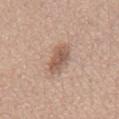subject = male, approximately 70 years of age | diameter = ~4.5 mm (longest diameter) | anatomic site = the front of the torso | image source = 15 mm crop, total-body photography | automated lesion analysis = a lesion area of about 8 mm², a shape eccentricity near 0.9, and a symmetry-axis asymmetry near 0.2; an average lesion color of about L≈56 a*≈19 b*≈27 (CIELAB) and roughly 11 lightness units darker than nearby skin.